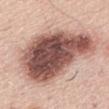biopsy_status: not biopsied; imaged during a skin examination
site: upper back
patient:
  sex: male
  age_approx: 60
lesion_size:
  long_diameter_mm_approx: 12.5
image:
  source: total-body photography crop
  field_of_view_mm: 15
automated_metrics:
  area_mm2_approx: 55.0
  shape_asymmetry: 0.3
  cielab_L: 53
  cielab_a: 21
  cielab_b: 24
  vs_skin_darker_L: 22.0
  vs_skin_contrast_norm: 13.5
  border_irregularity_0_10: 3.5
  color_variation_0_10: 9.5
  peripheral_color_asymmetry: 3.5
  nevus_likeness_0_100: 40
  lesion_detection_confidence_0_100: 100